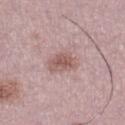Q: Was this lesion biopsied?
A: catalogued during a skin exam; not biopsied
Q: How was the tile lit?
A: white-light illumination
Q: Lesion location?
A: the left lower leg
Q: What is the imaging modality?
A: 15 mm crop, total-body photography
Q: Lesion size?
A: ~3 mm (longest diameter)
Q: Who is the patient?
A: male, aged approximately 50